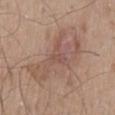Clinical impression:
Recorded during total-body skin imaging; not selected for excision or biopsy.
Clinical summary:
The lesion-visualizer software estimated a symmetry-axis asymmetry near 0.45. The software also gave an average lesion color of about L≈53 a*≈18 b*≈25 (CIELAB) and roughly 7 lightness units darker than nearby skin. About 8 mm across. Located on the back. A male subject, roughly 50 years of age. Imaged with white-light lighting. A region of skin cropped from a whole-body photographic capture, roughly 15 mm wide.